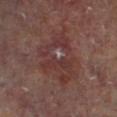notes: imaged on a skin check; not biopsied | acquisition: ~15 mm crop, total-body skin-cancer survey | lesion size: ≈7 mm | subject: male, aged 63–67 | site: the left lower leg.